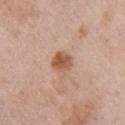Background:
About 2.5 mm across. From the arm. Imaged with white-light lighting. The patient is a female aged 63 to 67. A region of skin cropped from a whole-body photographic capture, roughly 15 mm wide. An algorithmic analysis of the crop reported a border-irregularity index near 1.5/10 and peripheral color asymmetry of about 1.5. The software also gave a classifier nevus-likeness of about 85/100 and lesion-presence confidence of about 100/100.